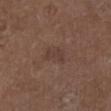No biopsy was performed on this lesion — it was imaged during a full skin examination and was not determined to be concerning.
Imaged with white-light lighting.
The recorded lesion diameter is about 3 mm.
A lesion tile, about 15 mm wide, cut from a 3D total-body photograph.
A male patient, roughly 75 years of age.
From the leg.
An algorithmic analysis of the crop reported a footprint of about 4 mm² and an eccentricity of roughly 0.85. It also reported a mean CIELAB color near L≈38 a*≈17 b*≈23, about 6 CIELAB-L* units darker than the surrounding skin, and a normalized lesion–skin contrast near 5.